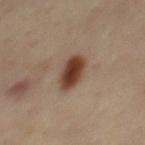No biopsy was performed on this lesion — it was imaged during a full skin examination and was not determined to be concerning. A region of skin cropped from a whole-body photographic capture, roughly 15 mm wide. The tile uses cross-polarized illumination. A male subject aged 43 to 47. Located on the mid back. About 4.5 mm across.